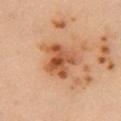follow-up: total-body-photography surveillance lesion; no biopsy
TBP lesion metrics: a border-irregularity index near 3.5/10, internal color variation of about 7.5 on a 0–10 scale, and a peripheral color-asymmetry measure near 2.5
subject: female, in their mid- to late 30s
image: ~15 mm tile from a whole-body skin photo
diameter: ≈4 mm
tile lighting: cross-polarized
body site: the front of the torso